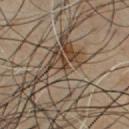Findings:
- biopsy status · imaged on a skin check; not biopsied
- imaging modality · 15 mm crop, total-body photography
- patient · male, aged 38–42
- site · the chest